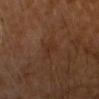Assessment:
This lesion was catalogued during total-body skin photography and was not selected for biopsy.
Acquisition and patient details:
This is a cross-polarized tile. Approximately 2.5 mm at its widest. The patient is a male approximately 70 years of age. On the head or neck. A 15 mm crop from a total-body photograph taken for skin-cancer surveillance.